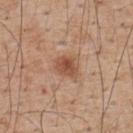Impression: The lesion was photographed on a routine skin check and not biopsied; there is no pathology result. Acquisition and patient details: A male subject, about 55 years old. This is a white-light tile. Cropped from a total-body skin-imaging series; the visible field is about 15 mm. About 3 mm across. On the upper back.